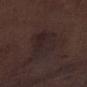workup=catalogued during a skin exam; not biopsied | subject=male, about 70 years old | image=~15 mm crop, total-body skin-cancer survey | location=the right lower leg.